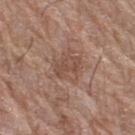The lesion was photographed on a routine skin check and not biopsied; there is no pathology result. Approximately 3 mm at its widest. The lesion is on the right thigh. A female subject, about 80 years old. A region of skin cropped from a whole-body photographic capture, roughly 15 mm wide.